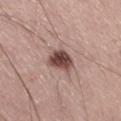biopsy status=total-body-photography surveillance lesion; no biopsy
illumination=white-light
imaging modality=total-body-photography crop, ~15 mm field of view
anatomic site=the right thigh
diameter=≈3 mm
patient=male, in their mid-50s